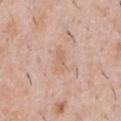biopsy status — catalogued during a skin exam; not biopsied
imaging modality — ~15 mm tile from a whole-body skin photo
subject — male, aged 38–42
tile lighting — white-light illumination
site — the abdomen